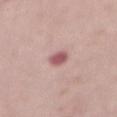Captured during whole-body skin photography for melanoma surveillance; the lesion was not biopsied. Longest diameter approximately 2.5 mm. A male subject, aged 68–72. The total-body-photography lesion software estimated a footprint of about 3.5 mm². It also reported a lesion color around L≈56 a*≈26 b*≈19 in CIELAB and a normalized border contrast of about 9. And it measured a border-irregularity rating of about 2/10, a within-lesion color-variation index near 2/10, and radial color variation of about 0.5. From the front of the torso. Imaged with white-light lighting. This image is a 15 mm lesion crop taken from a total-body photograph.